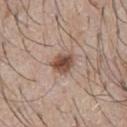notes = no biopsy performed (imaged during a skin exam); lighting = white-light illumination; subject = male, in their mid-60s; site = the chest; image source = ~15 mm crop, total-body skin-cancer survey; size = about 3 mm.